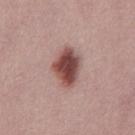<case>
  <lesion_size>
    <long_diameter_mm_approx>5.0</long_diameter_mm_approx>
  </lesion_size>
  <image>
    <source>total-body photography crop</source>
    <field_of_view_mm>15</field_of_view_mm>
  </image>
  <automated_metrics>
    <shape_asymmetry>0.1</shape_asymmetry>
  </automated_metrics>
  <lighting>white-light</lighting>
  <patient>
    <sex>male</sex>
    <age_approx>30</age_approx>
  </patient>
</case>